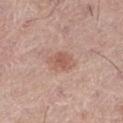Background:
The recorded lesion diameter is about 3.5 mm. Captured under white-light illumination. Located on the right thigh. A male patient aged 73–77. Cropped from a total-body skin-imaging series; the visible field is about 15 mm.